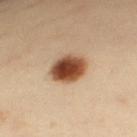Findings:
* workup · no biopsy performed (imaged during a skin exam)
* patient · female, in their 40s
* imaging modality · total-body-photography crop, ~15 mm field of view
* anatomic site · the left upper arm
* tile lighting · cross-polarized illumination
* diameter · about 4.5 mm